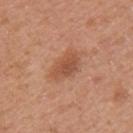Q: Was this lesion biopsied?
A: no biopsy performed (imaged during a skin exam)
Q: Lesion location?
A: the left upper arm
Q: What is the lesion's diameter?
A: ~4 mm (longest diameter)
Q: What is the imaging modality?
A: total-body-photography crop, ~15 mm field of view
Q: What are the patient's age and sex?
A: female, aged 38 to 42
Q: How was the tile lit?
A: white-light